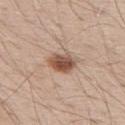Assessment:
The lesion was photographed on a routine skin check and not biopsied; there is no pathology result.
Image and clinical context:
The lesion is located on the upper back. A 15 mm close-up tile from a total-body photography series done for melanoma screening. Measured at roughly 3.5 mm in maximum diameter. Automated image analysis of the tile measured a border-irregularity rating of about 2.5/10 and internal color variation of about 5.5 on a 0–10 scale. A male patient, aged 68–72.